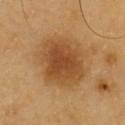A roughly 15 mm field-of-view crop from a total-body skin photograph. Measured at roughly 6 mm in maximum diameter. Automated tile analysis of the lesion measured a mean CIELAB color near L≈47 a*≈21 b*≈38 and a normalized border contrast of about 8. A male subject aged 58–62. The lesion is on the chest. The tile uses cross-polarized illumination.